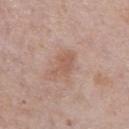The lesion was tiled from a total-body skin photograph and was not biopsied. A male patient aged 73–77. The recorded lesion diameter is about 3.5 mm. This image is a 15 mm lesion crop taken from a total-body photograph. On the chest.